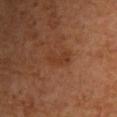| key | value |
|---|---|
| biopsy status | total-body-photography surveillance lesion; no biopsy |
| image | total-body-photography crop, ~15 mm field of view |
| automated metrics | a mean CIELAB color near L≈34 a*≈22 b*≈30, a lesion–skin lightness drop of about 5, and a lesion-to-skin contrast of about 5 (normalized; higher = more distinct) |
| lesion size | ≈3 mm |
| illumination | cross-polarized illumination |
| subject | female, approximately 60 years of age |
| site | the upper back |